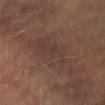Clinical impression:
This lesion was catalogued during total-body skin photography and was not selected for biopsy.
Background:
On the right lower leg. A lesion tile, about 15 mm wide, cut from a 3D total-body photograph. Captured under cross-polarized illumination. The subject is a male aged 58 to 62.